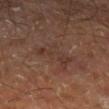{"biopsy_status": "not biopsied; imaged during a skin examination", "image": {"source": "total-body photography crop", "field_of_view_mm": 15}, "patient": {"sex": "male", "age_approx": 60}, "site": "right leg", "lesion_size": {"long_diameter_mm_approx": 4.5}, "lighting": "cross-polarized"}